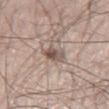No biopsy was performed on this lesion — it was imaged during a full skin examination and was not determined to be concerning. A close-up tile cropped from a whole-body skin photograph, about 15 mm across. About 3.5 mm across. The lesion is on the leg. Imaged with white-light lighting. A male subject roughly 60 years of age. Automated image analysis of the tile measured border irregularity of about 3 on a 0–10 scale, internal color variation of about 6.5 on a 0–10 scale, and a peripheral color-asymmetry measure near 2. The analysis additionally found a nevus-likeness score of about 10/100 and lesion-presence confidence of about 50/100.